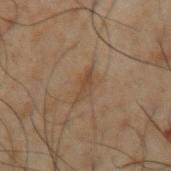Part of a total-body skin-imaging series; this lesion was reviewed on a skin check and was not flagged for biopsy. A 15 mm crop from a total-body photograph taken for skin-cancer surveillance. Captured under cross-polarized illumination. A male subject aged 48 to 52. Approximately 3 mm at its widest. The lesion-visualizer software estimated an average lesion color of about L≈33 a*≈13 b*≈24 (CIELAB), roughly 5 lightness units darker than nearby skin, and a lesion-to-skin contrast of about 6 (normalized; higher = more distinct). The analysis additionally found border irregularity of about 4.5 on a 0–10 scale and a peripheral color-asymmetry measure near 0. And it measured a classifier nevus-likeness of about 5/100 and lesion-presence confidence of about 100/100. Located on the left upper arm.